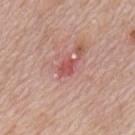Q: Was this lesion biopsied?
A: imaged on a skin check; not biopsied
Q: Lesion location?
A: the mid back
Q: Lesion size?
A: ~2.5 mm (longest diameter)
Q: What is the imaging modality?
A: ~15 mm crop, total-body skin-cancer survey
Q: Patient demographics?
A: male, aged 68 to 72